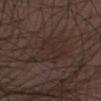Impression:
No biopsy was performed on this lesion — it was imaged during a full skin examination and was not determined to be concerning.
Acquisition and patient details:
The tile uses white-light illumination. A 15 mm crop from a total-body photograph taken for skin-cancer surveillance. A male patient, about 50 years old.